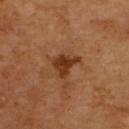The lesion was tiled from a total-body skin photograph and was not biopsied.
The lesion is on the back.
The tile uses cross-polarized illumination.
The lesion's longest dimension is about 3.5 mm.
The subject is a male about 60 years old.
The total-body-photography lesion software estimated a lesion area of about 6 mm² and two-axis asymmetry of about 0.5. The software also gave a border-irregularity index near 4.5/10, internal color variation of about 2.5 on a 0–10 scale, and radial color variation of about 1.
Cropped from a whole-body photographic skin survey; the tile spans about 15 mm.